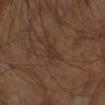  patient:
    sex: male
    age_approx: 60
  lighting: cross-polarized
  lesion_size:
    long_diameter_mm_approx: 2.5
  site: left arm
  image:
    source: total-body photography crop
    field_of_view_mm: 15